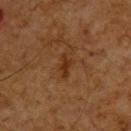Located on the upper back.
A male subject aged around 65.
A 15 mm close-up tile from a total-body photography series done for melanoma screening.